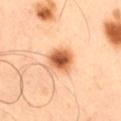Imaged during a routine full-body skin examination; the lesion was not biopsied and no histopathology is available. The tile uses cross-polarized illumination. A male patient, aged 48–52. A 15 mm close-up tile from a total-body photography series done for melanoma screening. Approximately 4 mm at its widest. Located on the right thigh.